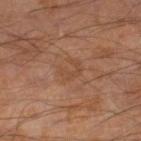notes=total-body-photography surveillance lesion; no biopsy | subject=male, roughly 65 years of age | body site=the right lower leg | image=15 mm crop, total-body photography.